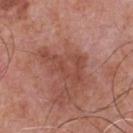| field | value |
|---|---|
| notes | imaged on a skin check; not biopsied |
| body site | the front of the torso |
| lighting | white-light |
| automated metrics | an outline eccentricity of about 0.85 (0 = round, 1 = elongated); internal color variation of about 1 on a 0–10 scale; an automated nevus-likeness rating near 0 out of 100 and lesion-presence confidence of about 100/100 |
| patient | male, about 70 years old |
| image | ~15 mm crop, total-body skin-cancer survey |
| lesion size | ~5.5 mm (longest diameter) |